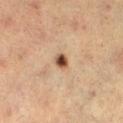The lesion is located on the leg. Automated tile analysis of the lesion measured an outline eccentricity of about 0.55 (0 = round, 1 = elongated). The analysis additionally found an average lesion color of about L≈39 a*≈17 b*≈27 (CIELAB), about 15 CIELAB-L* units darker than the surrounding skin, and a normalized border contrast of about 12. It also reported a border-irregularity rating of about 2/10, internal color variation of about 4 on a 0–10 scale, and radial color variation of about 1. Cropped from a whole-body photographic skin survey; the tile spans about 15 mm. Captured under cross-polarized illumination. The subject is a female aged 53–57. The biopsy diagnosis was a melanoma in situ, classified as a skin cancer.